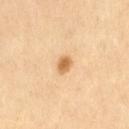{"biopsy_status": "not biopsied; imaged during a skin examination", "image": {"source": "total-body photography crop", "field_of_view_mm": 15}, "patient": {"sex": "female", "age_approx": 60}, "site": "abdomen", "lighting": "cross-polarized"}